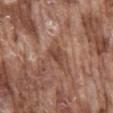| field | value |
|---|---|
| workup | imaged on a skin check; not biopsied |
| subject | male, in their mid- to late 70s |
| lighting | white-light |
| location | the mid back |
| imaging modality | total-body-photography crop, ~15 mm field of view |